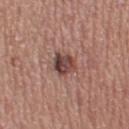The lesion was tiled from a total-body skin photograph and was not biopsied.
The lesion is located on the right thigh.
A female subject, roughly 45 years of age.
A close-up tile cropped from a whole-body skin photograph, about 15 mm across.
About 3 mm across.